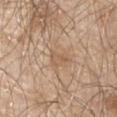workup: imaged on a skin check; not biopsied
TBP lesion metrics: a footprint of about 2.5 mm², a shape eccentricity near 0.85, and two-axis asymmetry of about 0.55; an automated nevus-likeness rating near 0 out of 100 and a lesion-detection confidence of about 95/100
image: total-body-photography crop, ~15 mm field of view
patient: male, in their mid- to late 60s
illumination: white-light
anatomic site: the left upper arm
lesion diameter: ~2.5 mm (longest diameter)